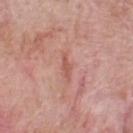Image and clinical context: Automated image analysis of the tile measured a mean CIELAB color near L≈56 a*≈26 b*≈28 and a lesion–skin lightness drop of about 8. The analysis additionally found a border-irregularity rating of about 4.5/10, internal color variation of about 0.5 on a 0–10 scale, and peripheral color asymmetry of about 0. This is a white-light tile. The recorded lesion diameter is about 3 mm. The lesion is located on the upper back. A region of skin cropped from a whole-body photographic capture, roughly 15 mm wide. A male subject aged around 60.